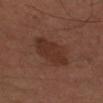follow-up: catalogued during a skin exam; not biopsied | lesion size: ≈5.5 mm | tile lighting: cross-polarized illumination | site: the left upper arm | subject: male, aged around 65 | image source: ~15 mm crop, total-body skin-cancer survey.